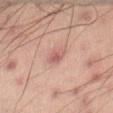Q: Was this lesion biopsied?
A: catalogued during a skin exam; not biopsied
Q: Who is the patient?
A: male, about 60 years old
Q: Lesion location?
A: the leg
Q: What lighting was used for the tile?
A: cross-polarized
Q: How was this image acquired?
A: ~15 mm tile from a whole-body skin photo
Q: What is the lesion's diameter?
A: about 2.5 mm